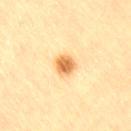No biopsy was performed on this lesion — it was imaged during a full skin examination and was not determined to be concerning. A male patient aged 83 or older. An algorithmic analysis of the crop reported a border-irregularity index near 1.5/10, a within-lesion color-variation index near 4/10, and a peripheral color-asymmetry measure near 1. It also reported an automated nevus-likeness rating near 100 out of 100 and a lesion-detection confidence of about 100/100. This image is a 15 mm lesion crop taken from a total-body photograph. The tile uses cross-polarized illumination. The lesion is located on the lower back.